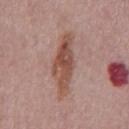Clinical impression:
No biopsy was performed on this lesion — it was imaged during a full skin examination and was not determined to be concerning.
Context:
Automated tile analysis of the lesion measured a border-irregularity index near 4/10. And it measured an automated nevus-likeness rating near 75 out of 100 and a lesion-detection confidence of about 100/100. A 15 mm close-up extracted from a 3D total-body photography capture. From the abdomen. Longest diameter approximately 7.5 mm. Captured under white-light illumination. The patient is a male roughly 75 years of age.